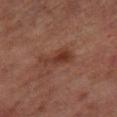Part of a total-body skin-imaging series; this lesion was reviewed on a skin check and was not flagged for biopsy. A female patient aged 58–62. Measured at roughly 4 mm in maximum diameter. A 15 mm close-up tile from a total-body photography series done for melanoma screening. This is a cross-polarized tile. The lesion is on the right thigh.